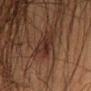The lesion was tiled from a total-body skin photograph and was not biopsied. A male subject, aged approximately 50. The lesion's longest dimension is about 4 mm. A 15 mm close-up tile from a total-body photography series done for melanoma screening. The lesion is located on the left upper arm.